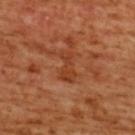notes = imaged on a skin check; not biopsied.